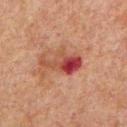Findings:
- biopsy status — catalogued during a skin exam; not biopsied
- patient — male, in their 60s
- anatomic site — the chest
- acquisition — 15 mm crop, total-body photography
- automated lesion analysis — a mean CIELAB color near L≈35 a*≈25 b*≈23, about 10 CIELAB-L* units darker than the surrounding skin, and a normalized border contrast of about 9.5
- lesion size — ~5 mm (longest diameter)